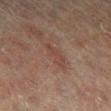Clinical impression:
This lesion was catalogued during total-body skin photography and was not selected for biopsy.
Context:
A female patient roughly 80 years of age. Approximately 4 mm at its widest. Automated image analysis of the tile measured a footprint of about 4 mm², a shape eccentricity near 0.95, and a shape-asymmetry score of about 0.3 (0 = symmetric). The software also gave a mean CIELAB color near L≈37 a*≈17 b*≈23, a lesion–skin lightness drop of about 5, and a normalized lesion–skin contrast near 5.5. And it measured border irregularity of about 4.5 on a 0–10 scale, a within-lesion color-variation index near 1/10, and peripheral color asymmetry of about 0. The analysis additionally found a detector confidence of about 95 out of 100 that the crop contains a lesion. Located on the right lower leg. A 15 mm crop from a total-body photograph taken for skin-cancer surveillance. This is a cross-polarized tile.